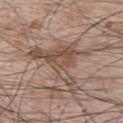Assessment: Imaged during a routine full-body skin examination; the lesion was not biopsied and no histopathology is available. Context: This image is a 15 mm lesion crop taken from a total-body photograph. The lesion's longest dimension is about 8.5 mm. The subject is a male in their mid- to late 60s. Captured under white-light illumination. On the upper back. The total-body-photography lesion software estimated a lesion area of about 20 mm², a shape eccentricity near 0.75, and a symmetry-axis asymmetry near 0.7. And it measured an average lesion color of about L≈50 a*≈16 b*≈25 (CIELAB), about 9 CIELAB-L* units darker than the surrounding skin, and a normalized border contrast of about 6.5. It also reported a border-irregularity index near 10/10 and a color-variation rating of about 5/10. The analysis additionally found a nevus-likeness score of about 0/100 and a detector confidence of about 55 out of 100 that the crop contains a lesion.